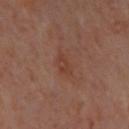Q: Patient demographics?
A: female, in their 50s
Q: Where on the body is the lesion?
A: the right upper arm
Q: What is the imaging modality?
A: ~15 mm crop, total-body skin-cancer survey